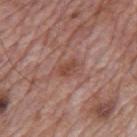Impression: Imaged during a routine full-body skin examination; the lesion was not biopsied and no histopathology is available. Context: From the mid back. Captured under white-light illumination. The recorded lesion diameter is about 2.5 mm. A roughly 15 mm field-of-view crop from a total-body skin photograph. A male subject approximately 70 years of age.